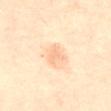<record>
<biopsy_status>not biopsied; imaged during a skin examination</biopsy_status>
</record>